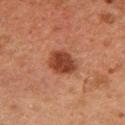Clinical summary:
The subject is a male aged 48 to 52. Located on the right upper arm. A region of skin cropped from a whole-body photographic capture, roughly 15 mm wide. About 3.5 mm across. An algorithmic analysis of the crop reported an area of roughly 8 mm², an eccentricity of roughly 0.55, and a symmetry-axis asymmetry near 0.2. And it measured roughly 11 lightness units darker than nearby skin and a normalized border contrast of about 10. The analysis additionally found a border-irregularity rating of about 2/10.